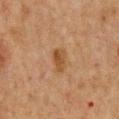Clinical impression: The lesion was tiled from a total-body skin photograph and was not biopsied. Image and clinical context: The tile uses cross-polarized illumination. Automated image analysis of the tile measured a lesion area of about 4.5 mm² and an outline eccentricity of about 0.85 (0 = round, 1 = elongated). The analysis additionally found an average lesion color of about L≈40 a*≈17 b*≈31 (CIELAB), a lesion–skin lightness drop of about 7, and a lesion-to-skin contrast of about 7 (normalized; higher = more distinct). A male subject, about 50 years old. The recorded lesion diameter is about 3 mm. A 15 mm close-up tile from a total-body photography series done for melanoma screening. The lesion is located on the chest.